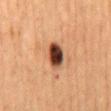Notes:
• biopsy status · no biopsy performed (imaged during a skin exam)
• automated lesion analysis · a lesion area of about 7 mm², an outline eccentricity of about 0.75 (0 = round, 1 = elongated), and two-axis asymmetry of about 0.25; border irregularity of about 2 on a 0–10 scale, internal color variation of about 6 on a 0–10 scale, and radial color variation of about 1.5
• patient · female, approximately 60 years of age
• acquisition · total-body-photography crop, ~15 mm field of view
• anatomic site · the mid back